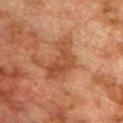The lesion was photographed on a routine skin check and not biopsied; there is no pathology result. Automated image analysis of the tile measured an average lesion color of about L≈40 a*≈22 b*≈31 (CIELAB) and a lesion–skin lightness drop of about 8. It also reported border irregularity of about 5.5 on a 0–10 scale and radial color variation of about 1.5. And it measured a classifier nevus-likeness of about 0/100. Measured at roughly 5.5 mm in maximum diameter. The lesion is on the chest. A male subject aged 73 to 77. Cropped from a whole-body photographic skin survey; the tile spans about 15 mm. The tile uses cross-polarized illumination.